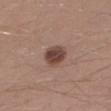biopsy status = total-body-photography surveillance lesion; no biopsy | image = total-body-photography crop, ~15 mm field of view | patient = male, aged around 30 | site = the left lower leg | illumination = white-light illumination | size = ≈3 mm.